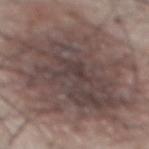notes: catalogued during a skin exam; not biopsied
patient: male, aged around 55
illumination: white-light
imaging modality: total-body-photography crop, ~15 mm field of view
body site: the abdomen
size: ~12 mm (longest diameter)
automated lesion analysis: a footprint of about 75 mm², an outline eccentricity of about 0.45 (0 = round, 1 = elongated), and a shape-asymmetry score of about 0.25 (0 = symmetric)